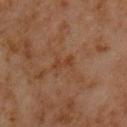Assessment:
No biopsy was performed on this lesion — it was imaged during a full skin examination and was not determined to be concerning.